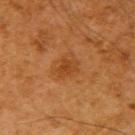On the right upper arm.
This image is a 15 mm lesion crop taken from a total-body photograph.
This is a cross-polarized tile.
A male subject, aged 58–62.
The lesion's longest dimension is about 2.5 mm.
Automated image analysis of the tile measured a lesion area of about 3.5 mm², a shape eccentricity near 0.7, and a shape-asymmetry score of about 0.35 (0 = symmetric). The software also gave a classifier nevus-likeness of about 40/100 and a detector confidence of about 100 out of 100 that the crop contains a lesion.